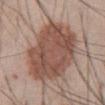Q: Was a biopsy performed?
A: no biopsy performed (imaged during a skin exam)
Q: How large is the lesion?
A: ≈8 mm
Q: Who is the patient?
A: male, aged approximately 45
Q: How was this image acquired?
A: ~15 mm tile from a whole-body skin photo
Q: Automated lesion metrics?
A: border irregularity of about 2 on a 0–10 scale and internal color variation of about 4 on a 0–10 scale
Q: Where on the body is the lesion?
A: the abdomen
Q: Illumination type?
A: white-light